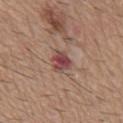Q: Was this lesion biopsied?
A: no biopsy performed (imaged during a skin exam)
Q: How large is the lesion?
A: about 3 mm
Q: How was the tile lit?
A: white-light
Q: How was this image acquired?
A: ~15 mm tile from a whole-body skin photo
Q: Patient demographics?
A: male, roughly 60 years of age
Q: Lesion location?
A: the mid back